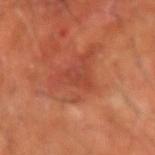Captured during whole-body skin photography for melanoma surveillance; the lesion was not biopsied. A male patient approximately 60 years of age. A lesion tile, about 15 mm wide, cut from a 3D total-body photograph. Located on the right forearm. Measured at roughly 3 mm in maximum diameter.